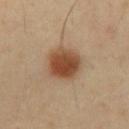biopsy status = total-body-photography surveillance lesion; no biopsy | tile lighting = cross-polarized illumination | imaging modality = ~15 mm tile from a whole-body skin photo | subject = male, in their mid- to late 50s | body site = the mid back | diameter = about 4.5 mm.